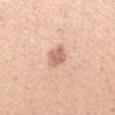biopsy status: total-body-photography surveillance lesion; no biopsy
lesion size: ~2.5 mm (longest diameter)
image-analysis metrics: a mean CIELAB color near L≈65 a*≈21 b*≈29, a lesion–skin lightness drop of about 12, and a normalized border contrast of about 7; lesion-presence confidence of about 100/100
patient: female, about 25 years old
acquisition: 15 mm crop, total-body photography
location: the left forearm
lighting: white-light illumination